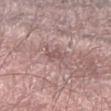Case summary:
• lighting: white-light illumination
• body site: the arm
• TBP lesion metrics: a footprint of about 3.5 mm² and two-axis asymmetry of about 0.3; about 8 CIELAB-L* units darker than the surrounding skin and a normalized border contrast of about 5.5
• imaging modality: ~15 mm tile from a whole-body skin photo
• size: ~2.5 mm (longest diameter)
• subject: male, roughly 65 years of age